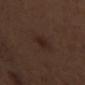Clinical impression:
Part of a total-body skin-imaging series; this lesion was reviewed on a skin check and was not flagged for biopsy.
Acquisition and patient details:
The lesion is on the arm. Cropped from a total-body skin-imaging series; the visible field is about 15 mm. The subject is a male aged 68 to 72. Automated image analysis of the tile measured an area of roughly 4 mm² and an outline eccentricity of about 0.8 (0 = round, 1 = elongated). And it measured a nevus-likeness score of about 85/100 and a lesion-detection confidence of about 100/100. Captured under white-light illumination. About 3 mm across.